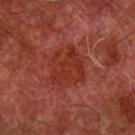Clinical impression: The lesion was photographed on a routine skin check and not biopsied; there is no pathology result. Image and clinical context: The lesion is located on the arm. This is a cross-polarized tile. An algorithmic analysis of the crop reported a border-irregularity rating of about 2/10, internal color variation of about 3 on a 0–10 scale, and radial color variation of about 1. The recorded lesion diameter is about 5 mm. A male subject aged 78 to 82. Cropped from a whole-body photographic skin survey; the tile spans about 15 mm.